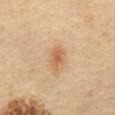No biopsy was performed on this lesion — it was imaged during a full skin examination and was not determined to be concerning.
The lesion is located on the abdomen.
A 15 mm close-up tile from a total-body photography series done for melanoma screening.
A male patient aged approximately 60.